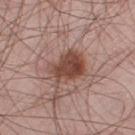The lesion was tiled from a total-body skin photograph and was not biopsied.